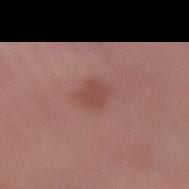Assessment:
Part of a total-body skin-imaging series; this lesion was reviewed on a skin check and was not flagged for biopsy.
Context:
From the right lower leg. A male patient in their mid- to late 60s. A 15 mm close-up extracted from a 3D total-body photography capture.